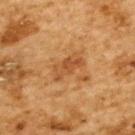No biopsy was performed on this lesion — it was imaged during a full skin examination and was not determined to be concerning. About 4 mm across. This is a cross-polarized tile. From the upper back. A 15 mm close-up extracted from a 3D total-body photography capture. A male patient, aged 83 or older. An algorithmic analysis of the crop reported a lesion area of about 6 mm², an outline eccentricity of about 0.9 (0 = round, 1 = elongated), and two-axis asymmetry of about 0.35. The software also gave a lesion-to-skin contrast of about 6.5 (normalized; higher = more distinct).